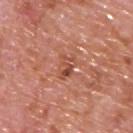The subject is a male aged approximately 65. On the back. The lesion-visualizer software estimated a shape eccentricity near 0.6 and a shape-asymmetry score of about 0.25 (0 = symmetric). The analysis additionally found an automated nevus-likeness rating near 0 out of 100 and lesion-presence confidence of about 100/100. Captured under white-light illumination. Cropped from a total-body skin-imaging series; the visible field is about 15 mm.